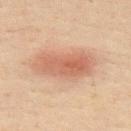This lesion was catalogued during total-body skin photography and was not selected for biopsy. A roughly 15 mm field-of-view crop from a total-body skin photograph. Located on the upper back. The tile uses cross-polarized illumination. A male patient aged 48 to 52.